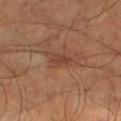{
  "biopsy_status": "not biopsied; imaged during a skin examination",
  "lighting": "cross-polarized",
  "image": {
    "source": "total-body photography crop",
    "field_of_view_mm": 15
  },
  "patient": {
    "sex": "male",
    "age_approx": 65
  },
  "site": "right lower leg",
  "lesion_size": {
    "long_diameter_mm_approx": 2.5
  },
  "automated_metrics": {
    "area_mm2_approx": 3.5,
    "eccentricity": 0.8,
    "shape_asymmetry": 0.35,
    "vs_skin_darker_L": 7.0,
    "vs_skin_contrast_norm": 6.0,
    "border_irregularity_0_10": 3.5,
    "color_variation_0_10": 1.0,
    "peripheral_color_asymmetry": 0.5
  }
}